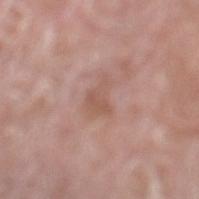The lesion was tiled from a total-body skin photograph and was not biopsied. The tile uses white-light illumination. A male subject, aged approximately 75. Located on the left lower leg. Automated tile analysis of the lesion measured radial color variation of about 0.5. The analysis additionally found a classifier nevus-likeness of about 0/100 and a detector confidence of about 100 out of 100 that the crop contains a lesion. A 15 mm close-up extracted from a 3D total-body photography capture. The lesion's longest dimension is about 3.5 mm.